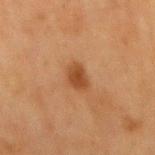The lesion was photographed on a routine skin check and not biopsied; there is no pathology result.
A male subject aged around 65.
Located on the mid back.
The total-body-photography lesion software estimated a footprint of about 5 mm² and a symmetry-axis asymmetry near 0.3. The software also gave a border-irregularity rating of about 3/10, internal color variation of about 2.5 on a 0–10 scale, and radial color variation of about 0.5.
A lesion tile, about 15 mm wide, cut from a 3D total-body photograph.
Captured under cross-polarized illumination.